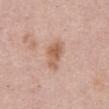Context: A female patient aged around 60. Imaged with white-light lighting. Approximately 4 mm at its widest. From the abdomen. A 15 mm crop from a total-body photograph taken for skin-cancer surveillance.